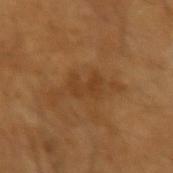notes = total-body-photography surveillance lesion; no biopsy | patient = male, aged 63 to 67 | acquisition = 15 mm crop, total-body photography | lesion diameter = ≈3.5 mm | illumination = cross-polarized.